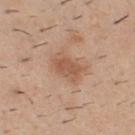notes: imaged on a skin check; not biopsied
tile lighting: white-light
subject: male, aged 38 to 42
automated metrics: a shape eccentricity near 0.75 and two-axis asymmetry of about 0.2; a mean CIELAB color near L≈56 a*≈20 b*≈31, roughly 9 lightness units darker than nearby skin, and a normalized lesion–skin contrast near 6.5; a classifier nevus-likeness of about 0/100 and lesion-presence confidence of about 100/100
image: ~15 mm crop, total-body skin-cancer survey
lesion size: ~4 mm (longest diameter)
location: the chest